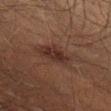workup: no biopsy performed (imaged during a skin exam) | body site: the abdomen | imaging modality: 15 mm crop, total-body photography | patient: male, aged approximately 45 | lighting: cross-polarized | image-analysis metrics: a lesion color around L≈22 a*≈16 b*≈19 in CIELAB, about 8 CIELAB-L* units darker than the surrounding skin, and a lesion-to-skin contrast of about 9.5 (normalized; higher = more distinct); border irregularity of about 3.5 on a 0–10 scale, a color-variation rating of about 1.5/10, and radial color variation of about 0.5; a lesion-detection confidence of about 100/100 | size: about 2.5 mm.